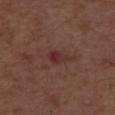Impression: No biopsy was performed on this lesion — it was imaged during a full skin examination and was not determined to be concerning. Context: The subject is a male roughly 50 years of age. Imaged with white-light lighting. An algorithmic analysis of the crop reported roughly 7 lightness units darker than nearby skin and a normalized lesion–skin contrast near 7. About 3 mm across. A close-up tile cropped from a whole-body skin photograph, about 15 mm across.